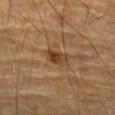The lesion's longest dimension is about 3 mm. From the left forearm. A male subject, aged approximately 85 or older. Automated image analysis of the tile measured a footprint of about 5 mm², a shape eccentricity near 0.8, and a symmetry-axis asymmetry near 0.25. The analysis additionally found border irregularity of about 2.5 on a 0–10 scale, a color-variation rating of about 5.5/10, and peripheral color asymmetry of about 2. This is a cross-polarized tile. A region of skin cropped from a whole-body photographic capture, roughly 15 mm wide.